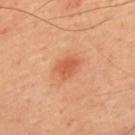Clinical impression: Captured during whole-body skin photography for melanoma surveillance; the lesion was not biopsied. Acquisition and patient details: About 2.5 mm across. A male subject about 60 years old. The lesion is located on the back. A region of skin cropped from a whole-body photographic capture, roughly 15 mm wide. An algorithmic analysis of the crop reported two-axis asymmetry of about 0.2. The analysis additionally found a border-irregularity index near 2/10 and a color-variation rating of about 2/10.